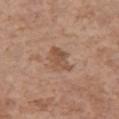No biopsy was performed on this lesion — it was imaged during a full skin examination and was not determined to be concerning. The subject is a female aged approximately 65. The lesion is on the left upper arm. A roughly 15 mm field-of-view crop from a total-body skin photograph.